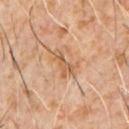{
  "biopsy_status": "not biopsied; imaged during a skin examination",
  "patient": {
    "sex": "male",
    "age_approx": 60
  },
  "image": {
    "source": "total-body photography crop",
    "field_of_view_mm": 15
  },
  "lesion_size": {
    "long_diameter_mm_approx": 4.0
  },
  "site": "chest"
}